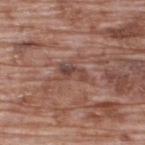<lesion>
<site>upper back</site>
<lighting>white-light</lighting>
<image>
  <source>total-body photography crop</source>
  <field_of_view_mm>15</field_of_view_mm>
</image>
<lesion_size>
  <long_diameter_mm_approx>3.0</long_diameter_mm_approx>
</lesion_size>
<patient>
  <sex>male</sex>
  <age_approx>70</age_approx>
</patient>
</lesion>